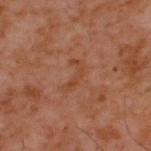Assessment: No biopsy was performed on this lesion — it was imaged during a full skin examination and was not determined to be concerning. Clinical summary: A male subject aged around 60. A roughly 15 mm field-of-view crop from a total-body skin photograph. From the upper back.